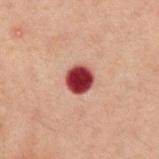Image and clinical context: Captured under cross-polarized illumination. An algorithmic analysis of the crop reported an average lesion color of about L≈32 a*≈28 b*≈21 (CIELAB) and a lesion-to-skin contrast of about 16.5 (normalized; higher = more distinct). It also reported an automated nevus-likeness rating near 0 out of 100. The lesion's longest dimension is about 3 mm. A male subject aged around 60. On the front of the torso. A region of skin cropped from a whole-body photographic capture, roughly 15 mm wide.